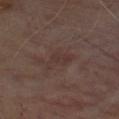Clinical impression: The lesion was photographed on a routine skin check and not biopsied; there is no pathology result. Acquisition and patient details: The total-body-photography lesion software estimated a lesion area of about 5 mm², an outline eccentricity of about 0.9 (0 = round, 1 = elongated), and two-axis asymmetry of about 0.5. And it measured an average lesion color of about L≈32 a*≈16 b*≈18 (CIELAB). And it measured a border-irregularity index near 5.5/10, internal color variation of about 2 on a 0–10 scale, and radial color variation of about 0.5. The tile uses cross-polarized illumination. A male patient about 70 years old. About 4 mm across. From the front of the torso. This image is a 15 mm lesion crop taken from a total-body photograph.